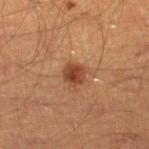Imaged during a routine full-body skin examination; the lesion was not biopsied and no histopathology is available.
A lesion tile, about 15 mm wide, cut from a 3D total-body photograph.
A male patient, in their mid- to late 40s.
From the right lower leg.